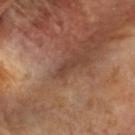Notes:
- workup · imaged on a skin check; not biopsied
- site · the head or neck
- acquisition · ~15 mm tile from a whole-body skin photo
- patient · female, roughly 55 years of age
- tile lighting · cross-polarized
- lesion diameter · ~2.5 mm (longest diameter)